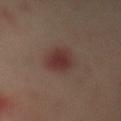{
  "biopsy_status": "not biopsied; imaged during a skin examination"
}